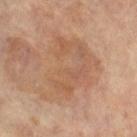Background:
The subject is a female aged around 65. The lesion is on the left leg. A region of skin cropped from a whole-body photographic capture, roughly 15 mm wide.